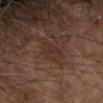follow-up: catalogued during a skin exam; not biopsied
patient: male, aged 63–67
imaging modality: ~15 mm tile from a whole-body skin photo
lighting: cross-polarized illumination
automated lesion analysis: an area of roughly 5.5 mm² and a shape-asymmetry score of about 0.3 (0 = symmetric); a mean CIELAB color near L≈29 a*≈17 b*≈21, roughly 5 lightness units darker than nearby skin, and a lesion-to-skin contrast of about 5 (normalized; higher = more distinct)
size: ≈3.5 mm
body site: the left forearm